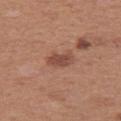No biopsy was performed on this lesion — it was imaged during a full skin examination and was not determined to be concerning. Located on the left thigh. Cropped from a total-body skin-imaging series; the visible field is about 15 mm. The patient is a female roughly 40 years of age. Automated tile analysis of the lesion measured an average lesion color of about L≈47 a*≈23 b*≈28 (CIELAB), about 10 CIELAB-L* units darker than the surrounding skin, and a lesion-to-skin contrast of about 7.5 (normalized; higher = more distinct). The analysis additionally found a classifier nevus-likeness of about 40/100 and lesion-presence confidence of about 100/100. Longest diameter approximately 3 mm. The tile uses white-light illumination.